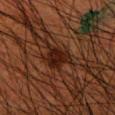Longest diameter approximately 3.5 mm.
A roughly 15 mm field-of-view crop from a total-body skin photograph.
An algorithmic analysis of the crop reported an area of roughly 7 mm² and a shape eccentricity near 0.65. It also reported a lesion color around L≈16 a*≈18 b*≈20 in CIELAB and a lesion-to-skin contrast of about 10.5 (normalized; higher = more distinct).
A male subject about 50 years old.
The lesion is located on the right forearm.
This is a cross-polarized tile.